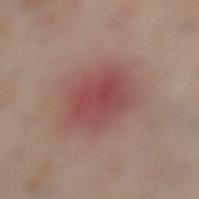Notes:
- follow-up · total-body-photography surveillance lesion; no biopsy
- acquisition · 15 mm crop, total-body photography
- patient · male, about 60 years old
- size · about 6 mm
- automated lesion analysis · a lesion color around L≈48 a*≈26 b*≈22 in CIELAB and a normalized border contrast of about 7; border irregularity of about 2 on a 0–10 scale and a color-variation rating of about 4.5/10
- body site · the left lower leg